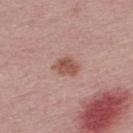Imaged with white-light lighting.
The lesion-visualizer software estimated an area of roughly 5.5 mm², a shape eccentricity near 0.65, and two-axis asymmetry of about 0.2. And it measured a lesion color around L≈53 a*≈23 b*≈27 in CIELAB, roughly 11 lightness units darker than nearby skin, and a lesion-to-skin contrast of about 8 (normalized; higher = more distinct). And it measured a nevus-likeness score of about 85/100 and a lesion-detection confidence of about 100/100.
A male patient, aged around 30.
A region of skin cropped from a whole-body photographic capture, roughly 15 mm wide.
The lesion's longest dimension is about 3 mm.